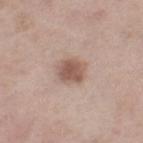subject: female, about 55 years old; image: total-body-photography crop, ~15 mm field of view; location: the left thigh.